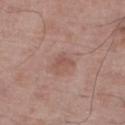notes = imaged on a skin check; not biopsied.